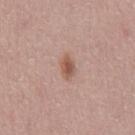{
  "biopsy_status": "not biopsied; imaged during a skin examination",
  "lesion_size": {
    "long_diameter_mm_approx": 3.0
  },
  "site": "chest",
  "patient": {
    "sex": "male",
    "age_approx": 45
  },
  "lighting": "white-light",
  "image": {
    "source": "total-body photography crop",
    "field_of_view_mm": 15
  },
  "automated_metrics": {
    "area_mm2_approx": 4.0,
    "shape_asymmetry": 0.2
  }
}